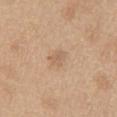– biopsy status · total-body-photography surveillance lesion; no biopsy
– anatomic site · the chest
– size · ~3 mm (longest diameter)
– illumination · white-light illumination
– imaging modality · ~15 mm tile from a whole-body skin photo
– TBP lesion metrics · a footprint of about 3.5 mm² and two-axis asymmetry of about 0.25; a mean CIELAB color near L≈61 a*≈17 b*≈33, roughly 7 lightness units darker than nearby skin, and a normalized border contrast of about 4.5
– subject · male, aged around 70